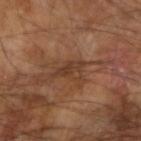<tbp_lesion>
<biopsy_status>not biopsied; imaged during a skin examination</biopsy_status>
<site>left arm</site>
<patient>
  <sex>male</sex>
  <age_approx>65</age_approx>
</patient>
<image>
  <source>total-body photography crop</source>
  <field_of_view_mm>15</field_of_view_mm>
</image>
</tbp_lesion>